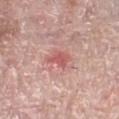<case>
<patient>
  <sex>female</sex>
  <age_approx>60</age_approx>
</patient>
<site>left lower leg</site>
<image>
  <source>total-body photography crop</source>
  <field_of_view_mm>15</field_of_view_mm>
</image>
</case>